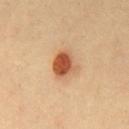This lesion was catalogued during total-body skin photography and was not selected for biopsy. A male patient, roughly 40 years of age. About 3.5 mm across. The lesion is on the chest. This image is a 15 mm lesion crop taken from a total-body photograph.